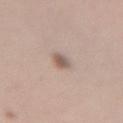Impression:
No biopsy was performed on this lesion — it was imaged during a full skin examination and was not determined to be concerning.
Image and clinical context:
Imaged with white-light lighting. The total-body-photography lesion software estimated an outline eccentricity of about 0.7 (0 = round, 1 = elongated) and two-axis asymmetry of about 0.25. And it measured a nevus-likeness score of about 95/100. Located on the left forearm. A roughly 15 mm field-of-view crop from a total-body skin photograph. The recorded lesion diameter is about 2.5 mm. A female subject, aged 23–27.